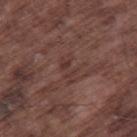A lesion tile, about 15 mm wide, cut from a 3D total-body photograph. Located on the right thigh. A male subject aged 73 to 77. About 3.5 mm across. This is a white-light tile.